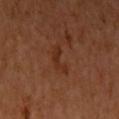The lesion was tiled from a total-body skin photograph and was not biopsied. A 15 mm crop from a total-body photograph taken for skin-cancer surveillance. Imaged with cross-polarized lighting. Approximately 3.5 mm at its widest. The lesion is on the left upper arm. A female subject, aged 38–42.